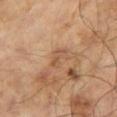| feature | finding |
|---|---|
| follow-up | catalogued during a skin exam; not biopsied |
| diameter | about 3 mm |
| subject | male, aged around 65 |
| automated metrics | border irregularity of about 4 on a 0–10 scale and internal color variation of about 0 on a 0–10 scale; lesion-presence confidence of about 95/100 |
| image | ~15 mm tile from a whole-body skin photo |